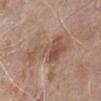No biopsy was performed on this lesion — it was imaged during a full skin examination and was not determined to be concerning. Approximately 7.5 mm at its widest. The lesion is on the right forearm. A region of skin cropped from a whole-body photographic capture, roughly 15 mm wide. The tile uses white-light illumination. A male patient in their mid- to late 70s. The lesion-visualizer software estimated a lesion color around L≈52 a*≈19 b*≈28 in CIELAB and a lesion-to-skin contrast of about 6.5 (normalized; higher = more distinct). The software also gave a border-irregularity index near 8/10, a color-variation rating of about 4/10, and peripheral color asymmetry of about 1.